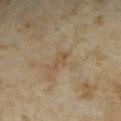notes: no biopsy performed (imaged during a skin exam); lesion diameter: about 3 mm; subject: female, approximately 35 years of age; anatomic site: the right upper arm; image: 15 mm crop, total-body photography; illumination: cross-polarized.